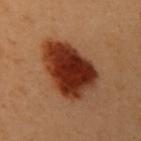Imaged during a routine full-body skin examination; the lesion was not biopsied and no histopathology is available.
A 15 mm crop from a total-body photograph taken for skin-cancer surveillance.
The tile uses cross-polarized illumination.
Approximately 7.5 mm at its widest.
From the chest.
The subject is a female aged approximately 60.